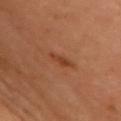No biopsy was performed on this lesion — it was imaged during a full skin examination and was not determined to be concerning.
This is a cross-polarized tile.
A female patient aged around 60.
A 15 mm crop from a total-body photograph taken for skin-cancer surveillance.
The recorded lesion diameter is about 3 mm.
On the chest.